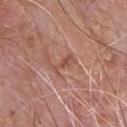• biopsy status — no biopsy performed (imaged during a skin exam)
• image source — 15 mm crop, total-body photography
• image-analysis metrics — a lesion area of about 3.5 mm², an outline eccentricity of about 0.9 (0 = round, 1 = elongated), and a shape-asymmetry score of about 0.4 (0 = symmetric); a lesion color around L≈51 a*≈23 b*≈28 in CIELAB and about 7 CIELAB-L* units darker than the surrounding skin; an automated nevus-likeness rating near 0 out of 100
• tile lighting — white-light illumination
• subject — male, roughly 60 years of age
• location — the front of the torso
• lesion size — ~3.5 mm (longest diameter)